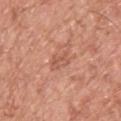Q: Patient demographics?
A: male, in their mid- to late 50s
Q: What is the imaging modality?
A: total-body-photography crop, ~15 mm field of view
Q: What is the anatomic site?
A: the back